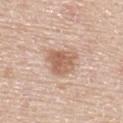Clinical impression:
No biopsy was performed on this lesion — it was imaged during a full skin examination and was not determined to be concerning.
Acquisition and patient details:
Automated tile analysis of the lesion measured a lesion area of about 9.5 mm², an eccentricity of roughly 0.35, and a shape-asymmetry score of about 0.25 (0 = symmetric). It also reported an average lesion color of about L≈61 a*≈20 b*≈30 (CIELAB). The software also gave border irregularity of about 3 on a 0–10 scale and a within-lesion color-variation index near 4/10. The analysis additionally found a classifier nevus-likeness of about 90/100 and a detector confidence of about 100 out of 100 that the crop contains a lesion. On the upper back. This is a white-light tile. The lesion's longest dimension is about 3.5 mm. A male subject approximately 60 years of age. A roughly 15 mm field-of-view crop from a total-body skin photograph.